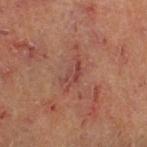The lesion was photographed on a routine skin check and not biopsied; there is no pathology result. From the left thigh. The recorded lesion diameter is about 3.5 mm. Imaged with cross-polarized lighting. A male subject, approximately 65 years of age. Cropped from a total-body skin-imaging series; the visible field is about 15 mm.